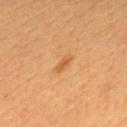Captured during whole-body skin photography for melanoma surveillance; the lesion was not biopsied.
A male subject, aged around 60.
Cropped from a whole-body photographic skin survey; the tile spans about 15 mm.
From the upper back.